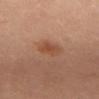Notes:
• follow-up — catalogued during a skin exam; not biopsied
• site — the front of the torso
• lesion diameter — ~2.5 mm (longest diameter)
• subject — female, roughly 30 years of age
• automated lesion analysis — a border-irregularity rating of about 2.5/10, a within-lesion color-variation index near 3.5/10, and radial color variation of about 1.5; an automated nevus-likeness rating near 50 out of 100 and lesion-presence confidence of about 100/100
• acquisition — total-body-photography crop, ~15 mm field of view